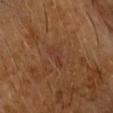Acquisition and patient details:
Measured at roughly 3.5 mm in maximum diameter. A male subject aged 68–72. Automated tile analysis of the lesion measured a border-irregularity index near 5.5/10 and internal color variation of about 0.5 on a 0–10 scale. And it measured a detector confidence of about 80 out of 100 that the crop contains a lesion. Imaged with cross-polarized lighting. On the head or neck. A close-up tile cropped from a whole-body skin photograph, about 15 mm across.